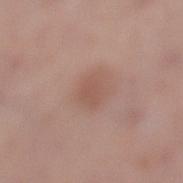Case summary:
• biopsy status — catalogued during a skin exam; not biopsied
• image-analysis metrics — an eccentricity of roughly 0.3 and a symmetry-axis asymmetry near 0.2; a lesion–skin lightness drop of about 7 and a normalized border contrast of about 5; a border-irregularity rating of about 2/10, a color-variation rating of about 1.5/10, and radial color variation of about 0.5
• imaging modality — ~15 mm crop, total-body skin-cancer survey
• tile lighting — white-light illumination
• size — ≈2.5 mm
• site — the left lower leg
• subject — female, in their 50s